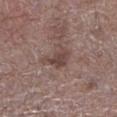Assessment:
Captured during whole-body skin photography for melanoma surveillance; the lesion was not biopsied.
Clinical summary:
A male patient, about 70 years old. A 15 mm close-up extracted from a 3D total-body photography capture. The lesion is located on the leg.